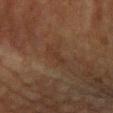biopsy status = catalogued during a skin exam; not biopsied.